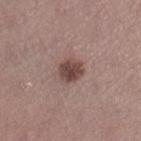Impression:
Captured during whole-body skin photography for melanoma surveillance; the lesion was not biopsied.
Background:
The lesion-visualizer software estimated an average lesion color of about L≈45 a*≈19 b*≈21 (CIELAB), roughly 13 lightness units darker than nearby skin, and a normalized lesion–skin contrast near 9.5. And it measured a nevus-likeness score of about 85/100 and a detector confidence of about 100 out of 100 that the crop contains a lesion. A 15 mm crop from a total-body photograph taken for skin-cancer surveillance. This is a white-light tile. The patient is a female aged 28–32. The lesion is on the right lower leg.